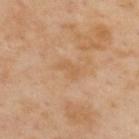{
  "biopsy_status": "not biopsied; imaged during a skin examination",
  "lesion_size": {
    "long_diameter_mm_approx": 3.0
  },
  "image": {
    "source": "total-body photography crop",
    "field_of_view_mm": 15
  },
  "patient": {
    "sex": "male",
    "age_approx": 55
  },
  "site": "back"
}